A roughly 15 mm field-of-view crop from a total-body skin photograph; the patient is aged around 60; on the left leg.
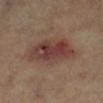Captured under cross-polarized illumination. The lesion's longest dimension is about 6 mm. The lesion-visualizer software estimated a shape eccentricity near 0.75 and two-axis asymmetry of about 0.2. It also reported a detector confidence of about 100 out of 100 that the crop contains a lesion. The lesion was biopsied, and histopathology showed a superficial basal cell carcinoma (malignant).